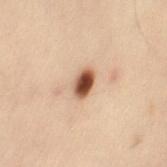* notes: no biopsy performed (imaged during a skin exam)
* tile lighting: cross-polarized
* imaging modality: ~15 mm crop, total-body skin-cancer survey
* TBP lesion metrics: a lesion color around L≈46 a*≈18 b*≈28 in CIELAB, about 18 CIELAB-L* units darker than the surrounding skin, and a normalized border contrast of about 12.5
* patient: female, aged 48–52
* diameter: about 3 mm
* body site: the left thigh